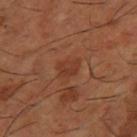Imaged during a routine full-body skin examination; the lesion was not biopsied and no histopathology is available.
An algorithmic analysis of the crop reported an area of roughly 4 mm². The analysis additionally found an average lesion color of about L≈37 a*≈25 b*≈31 (CIELAB) and a lesion–skin lightness drop of about 6. The analysis additionally found a border-irregularity index near 2.5/10, internal color variation of about 2 on a 0–10 scale, and a peripheral color-asymmetry measure near 1. The analysis additionally found a classifier nevus-likeness of about 0/100 and lesion-presence confidence of about 100/100.
Cropped from a total-body skin-imaging series; the visible field is about 15 mm.
A male patient, roughly 65 years of age.
Approximately 2.5 mm at its widest.
From the right thigh.